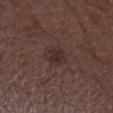Q: Is there a histopathology result?
A: no biopsy performed (imaged during a skin exam)
Q: Patient demographics?
A: male, roughly 50 years of age
Q: What is the anatomic site?
A: the left forearm
Q: What kind of image is this?
A: ~15 mm tile from a whole-body skin photo